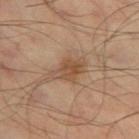Part of a total-body skin-imaging series; this lesion was reviewed on a skin check and was not flagged for biopsy.
A male subject, aged approximately 45.
Automated tile analysis of the lesion measured a lesion area of about 6.5 mm², an eccentricity of roughly 0.8, and two-axis asymmetry of about 0.3. The software also gave a lesion color around L≈49 a*≈18 b*≈31 in CIELAB and roughly 9 lightness units darker than nearby skin. The software also gave a color-variation rating of about 3.5/10 and peripheral color asymmetry of about 1. The analysis additionally found a nevus-likeness score of about 50/100 and lesion-presence confidence of about 100/100.
A close-up tile cropped from a whole-body skin photograph, about 15 mm across.
From the left thigh.
Imaged with cross-polarized lighting.